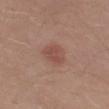Q: Was this lesion biopsied?
A: imaged on a skin check; not biopsied
Q: Who is the patient?
A: male, aged approximately 30
Q: What kind of image is this?
A: ~15 mm crop, total-body skin-cancer survey
Q: What lighting was used for the tile?
A: white-light
Q: Where on the body is the lesion?
A: the leg
Q: What did automated image analysis measure?
A: a border-irregularity rating of about 2.5/10 and a within-lesion color-variation index near 1.5/10
Q: What is the lesion's diameter?
A: ≈3 mm